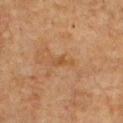biopsy status: imaged on a skin check; not biopsied.